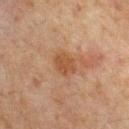biopsy status: catalogued during a skin exam; not biopsied
body site: the back
automated metrics: a border-irregularity index near 2/10 and internal color variation of about 2 on a 0–10 scale; a classifier nevus-likeness of about 5/100 and lesion-presence confidence of about 100/100
patient: male, in their mid- to late 60s
acquisition: ~15 mm crop, total-body skin-cancer survey
lighting: cross-polarized
size: ~3 mm (longest diameter)